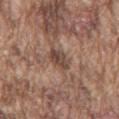Q: Was a biopsy performed?
A: imaged on a skin check; not biopsied
Q: How was the tile lit?
A: white-light
Q: What are the patient's age and sex?
A: male, in their mid- to late 70s
Q: What is the anatomic site?
A: the mid back
Q: What did automated image analysis measure?
A: an outline eccentricity of about 0.75 (0 = round, 1 = elongated) and a symmetry-axis asymmetry near 0.25; a mean CIELAB color near L≈44 a*≈17 b*≈24, a lesion–skin lightness drop of about 11, and a normalized lesion–skin contrast near 8.5; a border-irregularity rating of about 2.5/10, a color-variation rating of about 4.5/10, and a peripheral color-asymmetry measure near 1.5; lesion-presence confidence of about 95/100
Q: What is the lesion's diameter?
A: ≈3 mm
Q: What is the imaging modality?
A: ~15 mm crop, total-body skin-cancer survey